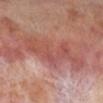Findings:
- notes: no biopsy performed (imaged during a skin exam)
- tile lighting: cross-polarized
- location: the right lower leg
- subject: male, approximately 70 years of age
- imaging modality: ~15 mm tile from a whole-body skin photo
- diameter: ~8 mm (longest diameter)
- automated lesion analysis: an area of roughly 17 mm², a shape eccentricity near 0.9, and a shape-asymmetry score of about 0.6 (0 = symmetric); a within-lesion color-variation index near 4.5/10